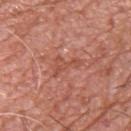This lesion was catalogued during total-body skin photography and was not selected for biopsy.
A male subject roughly 55 years of age.
About 4 mm across.
A lesion tile, about 15 mm wide, cut from a 3D total-body photograph.
The lesion-visualizer software estimated a footprint of about 4 mm², an eccentricity of roughly 0.85, and a shape-asymmetry score of about 0.8 (0 = symmetric). It also reported a lesion color around L≈51 a*≈28 b*≈31 in CIELAB, a lesion–skin lightness drop of about 7, and a normalized border contrast of about 5. The software also gave border irregularity of about 9.5 on a 0–10 scale and a within-lesion color-variation index near 0/10. And it measured an automated nevus-likeness rating near 0 out of 100 and a lesion-detection confidence of about 75/100.
On the upper back.
Captured under white-light illumination.